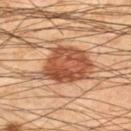This lesion was catalogued during total-body skin photography and was not selected for biopsy.